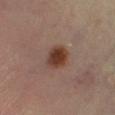No biopsy was performed on this lesion — it was imaged during a full skin examination and was not determined to be concerning. A male patient about 55 years old. Imaged with cross-polarized lighting. Automated tile analysis of the lesion measured a color-variation rating of about 4/10 and peripheral color asymmetry of about 1. The software also gave a nevus-likeness score of about 100/100 and lesion-presence confidence of about 100/100. This image is a 15 mm lesion crop taken from a total-body photograph. About 3.5 mm across. Located on the leg.